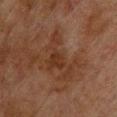Impression:
No biopsy was performed on this lesion — it was imaged during a full skin examination and was not determined to be concerning.
Context:
The patient is a male in their mid- to late 70s. From the upper back. A 15 mm close-up extracted from a 3D total-body photography capture.